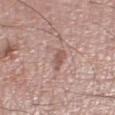{"biopsy_status": "not biopsied; imaged during a skin examination", "site": "right lower leg", "patient": {"sex": "male", "age_approx": 70}, "automated_metrics": {"eccentricity": 0.85, "shape_asymmetry": 0.6, "cielab_L": 57, "cielab_a": 19, "cielab_b": 23, "vs_skin_darker_L": 8.0, "lesion_detection_confidence_0_100": 95}, "image": {"source": "total-body photography crop", "field_of_view_mm": 15}}